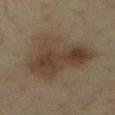Clinical impression: Recorded during total-body skin imaging; not selected for excision or biopsy. Acquisition and patient details: Approximately 7.5 mm at its widest. The subject is a male aged approximately 35. The lesion-visualizer software estimated a lesion area of about 34 mm² and a shape eccentricity near 0.5. And it measured a mean CIELAB color near L≈36 a*≈12 b*≈23, a lesion–skin lightness drop of about 8, and a lesion-to-skin contrast of about 8 (normalized; higher = more distinct). A roughly 15 mm field-of-view crop from a total-body skin photograph. The lesion is located on the abdomen. The tile uses cross-polarized illumination.